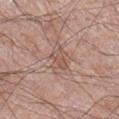<case>
<biopsy_status>not biopsied; imaged during a skin examination</biopsy_status>
<site>right lower leg</site>
<lesion_size>
  <long_diameter_mm_approx>3.0</long_diameter_mm_approx>
</lesion_size>
<lighting>white-light</lighting>
<image>
  <source>total-body photography crop</source>
  <field_of_view_mm>15</field_of_view_mm>
</image>
<patient>
  <sex>male</sex>
  <age_approx>60</age_approx>
</patient>
</case>